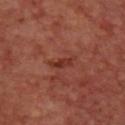workup = total-body-photography surveillance lesion; no biopsy | patient = female, approximately 45 years of age | body site = the upper back | acquisition = total-body-photography crop, ~15 mm field of view.